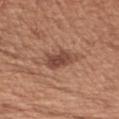<case>
  <biopsy_status>not biopsied; imaged during a skin examination</biopsy_status>
  <site>left upper arm</site>
  <image>
    <source>total-body photography crop</source>
    <field_of_view_mm>15</field_of_view_mm>
  </image>
  <patient>
    <sex>female</sex>
    <age_approx>40</age_approx>
  </patient>
  <automated_metrics>
    <area_mm2_approx>7.5</area_mm2_approx>
    <eccentricity>0.8</eccentricity>
    <shape_asymmetry>0.25</shape_asymmetry>
    <cielab_L>46</cielab_L>
    <cielab_a>22</cielab_a>
    <cielab_b>27</cielab_b>
    <vs_skin_darker_L>11.0</vs_skin_darker_L>
    <vs_skin_contrast_norm>8.5</vs_skin_contrast_norm>
    <nevus_likeness_0_100>70</nevus_likeness_0_100>
  </automated_metrics>
  <lighting>white-light</lighting>
  <lesion_size>
    <long_diameter_mm_approx>4.0</long_diameter_mm_approx>
  </lesion_size>
</case>